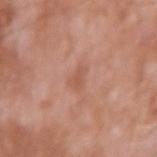illumination — white-light illumination
location — the right upper arm
patient — male, approximately 60 years of age
imaging modality — ~15 mm tile from a whole-body skin photo
automated metrics — a lesion area of about 4 mm² and an eccentricity of roughly 0.7; border irregularity of about 3 on a 0–10 scale and a peripheral color-asymmetry measure near 0.5; a nevus-likeness score of about 0/100 and a lesion-detection confidence of about 100/100